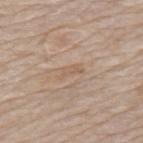The tile uses white-light illumination. A close-up tile cropped from a whole-body skin photograph, about 15 mm across. The recorded lesion diameter is about 3 mm. Located on the mid back. An algorithmic analysis of the crop reported an average lesion color of about L≈58 a*≈16 b*≈30 (CIELAB), a lesion–skin lightness drop of about 6, and a lesion-to-skin contrast of about 5 (normalized; higher = more distinct). A male patient, aged 73 to 77.